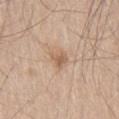No biopsy was performed on this lesion — it was imaged during a full skin examination and was not determined to be concerning. Cropped from a total-body skin-imaging series; the visible field is about 15 mm. The total-body-photography lesion software estimated a footprint of about 4 mm², an outline eccentricity of about 0.6 (0 = round, 1 = elongated), and two-axis asymmetry of about 0.4. And it measured an average lesion color of about L≈60 a*≈18 b*≈32 (CIELAB), roughly 9 lightness units darker than nearby skin, and a normalized lesion–skin contrast near 6.5. And it measured internal color variation of about 3 on a 0–10 scale and peripheral color asymmetry of about 1. The patient is a male aged 63–67. The lesion is located on the right thigh.